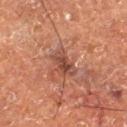Q: Was this lesion biopsied?
A: catalogued during a skin exam; not biopsied
Q: Lesion size?
A: about 4 mm
Q: Who is the patient?
A: male, aged 73–77
Q: What lighting was used for the tile?
A: cross-polarized
Q: What kind of image is this?
A: ~15 mm tile from a whole-body skin photo
Q: Where on the body is the lesion?
A: the right thigh
Q: Automated lesion metrics?
A: a footprint of about 6.5 mm² and a shape-asymmetry score of about 0.4 (0 = symmetric); a nevus-likeness score of about 0/100 and a detector confidence of about 95 out of 100 that the crop contains a lesion